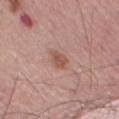The recorded lesion diameter is about 2.5 mm. The lesion is located on the left thigh. A 15 mm close-up extracted from a 3D total-body photography capture. The lesion-visualizer software estimated an area of roughly 4 mm², an outline eccentricity of about 0.7 (0 = round, 1 = elongated), and two-axis asymmetry of about 0.25. It also reported a mean CIELAB color near L≈54 a*≈22 b*≈27 and a lesion-to-skin contrast of about 7 (normalized; higher = more distinct). The subject is a male aged 68–72. This is a white-light tile.